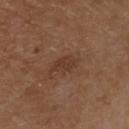image source — 15 mm crop, total-body photography | anatomic site — the front of the torso | lesion diameter — about 3.5 mm | subject — male, roughly 70 years of age.